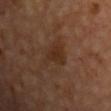The lesion was tiled from a total-body skin photograph and was not biopsied.
The lesion's longest dimension is about 4 mm.
The lesion is located on the chest.
Captured under cross-polarized illumination.
A region of skin cropped from a whole-body photographic capture, roughly 15 mm wide.
The subject is in their mid- to late 60s.